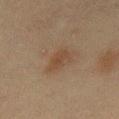<lesion>
  <biopsy_status>not biopsied; imaged during a skin examination</biopsy_status>
  <image>
    <source>total-body photography crop</source>
    <field_of_view_mm>15</field_of_view_mm>
  </image>
  <lesion_size>
    <long_diameter_mm_approx>3.0</long_diameter_mm_approx>
  </lesion_size>
  <lighting>cross-polarized</lighting>
  <site>chest</site>
  <automated_metrics>
    <area_mm2_approx>4.0</area_mm2_approx>
    <shape_asymmetry>0.25</shape_asymmetry>
    <vs_skin_darker_L>6.0</vs_skin_darker_L>
    <vs_skin_contrast_norm>6.0</vs_skin_contrast_norm>
    <border_irregularity_0_10>2.5</border_irregularity_0_10>
    <color_variation_0_10>1.0</color_variation_0_10>
    <peripheral_color_asymmetry>0.5</peripheral_color_asymmetry>
  </automated_metrics>
  <patient>
    <sex>male</sex>
    <age_approx>45</age_approx>
  </patient>
</lesion>